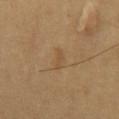Captured under cross-polarized illumination.
On the chest.
A male patient, aged 63 to 67.
Longest diameter approximately 2.5 mm.
A close-up tile cropped from a whole-body skin photograph, about 15 mm across.